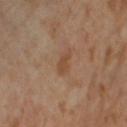workup = catalogued during a skin exam; not biopsied
subject = female, aged 53–57
automated metrics = a lesion–skin lightness drop of about 7; a lesion-detection confidence of about 100/100
body site = the leg
illumination = cross-polarized illumination
image source = total-body-photography crop, ~15 mm field of view
size = about 3 mm